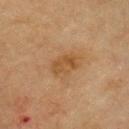  biopsy_status: not biopsied; imaged during a skin examination
  patient:
    sex: male
    age_approx: 70
  site: chest
  lesion_size:
    long_diameter_mm_approx: 3.5
  image:
    source: total-body photography crop
    field_of_view_mm: 15
  automated_metrics:
    area_mm2_approx: 6.0
    nevus_likeness_0_100: 5
    lesion_detection_confidence_0_100: 100